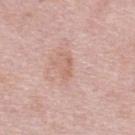Clinical impression:
Captured during whole-body skin photography for melanoma surveillance; the lesion was not biopsied.
Context:
A 15 mm crop from a total-body photograph taken for skin-cancer surveillance. Imaged with white-light lighting. Located on the upper back. About 2.5 mm across. The subject is a female roughly 65 years of age.